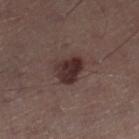Q: Was this lesion biopsied?
A: total-body-photography surveillance lesion; no biopsy
Q: How large is the lesion?
A: ~3.5 mm (longest diameter)
Q: Who is the patient?
A: male, approximately 55 years of age
Q: What kind of image is this?
A: ~15 mm crop, total-body skin-cancer survey
Q: Illumination type?
A: white-light illumination
Q: Where on the body is the lesion?
A: the right lower leg